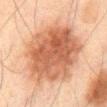notes — no biopsy performed (imaged during a skin exam)
imaging modality — total-body-photography crop, ~15 mm field of view
size — about 9 mm
location — the lower back
TBP lesion metrics — a footprint of about 50 mm²; border irregularity of about 2 on a 0–10 scale, a within-lesion color-variation index near 5.5/10, and radial color variation of about 1.5; a classifier nevus-likeness of about 70/100 and a lesion-detection confidence of about 100/100
illumination — cross-polarized
patient — male, about 65 years old